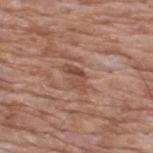Cropped from a total-body skin-imaging series; the visible field is about 15 mm. Imaged with white-light lighting. The lesion is located on the upper back. Approximately 3.5 mm at its widest. The patient is a male aged around 60.